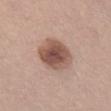workup=no biopsy performed (imaged during a skin exam) | imaging modality=total-body-photography crop, ~15 mm field of view | anatomic site=the front of the torso | patient=female, aged around 25.